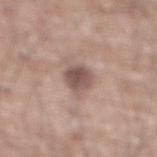biopsy_status: not biopsied; imaged during a skin examination
image:
  source: total-body photography crop
  field_of_view_mm: 15
automated_metrics:
  area_mm2_approx: 6.0
  eccentricity: 0.6
  nevus_likeness_0_100: 10
  lesion_detection_confidence_0_100: 100
lighting: white-light
patient:
  sex: male
  age_approx: 75
site: abdomen